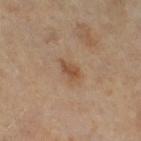{
  "biopsy_status": "not biopsied; imaged during a skin examination",
  "site": "right thigh",
  "lesion_size": {
    "long_diameter_mm_approx": 3.0
  },
  "image": {
    "source": "total-body photography crop",
    "field_of_view_mm": 15
  },
  "patient": {
    "sex": "female",
    "age_approx": 50
  },
  "lighting": "cross-polarized"
}